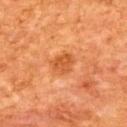This image is a 15 mm lesion crop taken from a total-body photograph. The patient is a male in their mid-60s. Captured under cross-polarized illumination. The lesion is on the upper back.